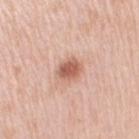<record>
<biopsy_status>not biopsied; imaged during a skin examination</biopsy_status>
</record>